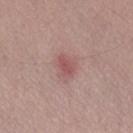This lesion was catalogued during total-body skin photography and was not selected for biopsy. Longest diameter approximately 2.5 mm. A 15 mm crop from a total-body photograph taken for skin-cancer surveillance. The patient is a male about 55 years old. The lesion is located on the left forearm. The lesion-visualizer software estimated a border-irregularity rating of about 2/10, a color-variation rating of about 1.5/10, and radial color variation of about 0.5. It also reported a classifier nevus-likeness of about 5/100 and a lesion-detection confidence of about 100/100. This is a white-light tile.